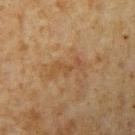The lesion was tiled from a total-body skin photograph and was not biopsied.
A male patient, aged approximately 60.
The lesion-visualizer software estimated an average lesion color of about L≈40 a*≈17 b*≈31 (CIELAB) and roughly 5 lightness units darker than nearby skin.
Approximately 4 mm at its widest.
The tile uses cross-polarized illumination.
A roughly 15 mm field-of-view crop from a total-body skin photograph.
The lesion is located on the left upper arm.